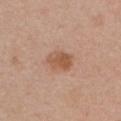This lesion was catalogued during total-body skin photography and was not selected for biopsy.
The patient is a female aged 53 to 57.
A close-up tile cropped from a whole-body skin photograph, about 15 mm across.
Located on the chest.
This is a white-light tile.
About 3.5 mm across.